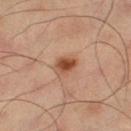Notes:
* biopsy status — imaged on a skin check; not biopsied
* site — the right thigh
* diameter — ~3 mm (longest diameter)
* automated metrics — a lesion-detection confidence of about 100/100
* patient — male, aged around 60
* acquisition — 15 mm crop, total-body photography